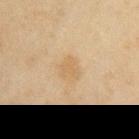Q: How was the tile lit?
A: cross-polarized
Q: How was this image acquired?
A: 15 mm crop, total-body photography
Q: What is the anatomic site?
A: the right upper arm
Q: Who is the patient?
A: male, aged 43 to 47